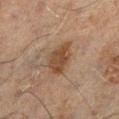The lesion was photographed on a routine skin check and not biopsied; there is no pathology result.
A region of skin cropped from a whole-body photographic capture, roughly 15 mm wide.
Longest diameter approximately 4 mm.
Automated tile analysis of the lesion measured about 8 CIELAB-L* units darker than the surrounding skin and a normalized border contrast of about 8. And it measured a border-irregularity index near 2.5/10 and internal color variation of about 2.5 on a 0–10 scale. The software also gave an automated nevus-likeness rating near 75 out of 100 and lesion-presence confidence of about 100/100.
Located on the left lower leg.
A male patient aged 58 to 62.